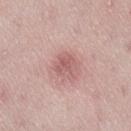The lesion's longest dimension is about 3 mm. The total-body-photography lesion software estimated a lesion color around L≈58 a*≈25 b*≈22 in CIELAB. And it measured a nevus-likeness score of about 0/100 and a detector confidence of about 100 out of 100 that the crop contains a lesion. A 15 mm crop from a total-body photograph taken for skin-cancer surveillance. A female subject approximately 40 years of age. The tile uses white-light illumination. From the lower back.